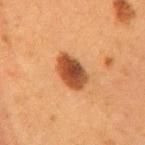{"lighting": "cross-polarized", "image": {"source": "total-body photography crop", "field_of_view_mm": 15}, "patient": {"sex": "female", "age_approx": 50}, "lesion_size": {"long_diameter_mm_approx": 4.5}, "automated_metrics": {"cielab_L": 40, "cielab_a": 23, "cielab_b": 33, "vs_skin_darker_L": 15.0, "vs_skin_contrast_norm": 11.5}, "site": "mid back"}